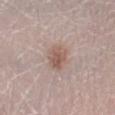Case summary:
- workup · catalogued during a skin exam; not biopsied
- tile lighting · white-light
- lesion size · ≈3.5 mm
- site · the right lower leg
- patient · female, aged 28–32
- TBP lesion metrics · a lesion area of about 7.5 mm², an outline eccentricity of about 0.65 (0 = round, 1 = elongated), and a shape-asymmetry score of about 0.2 (0 = symmetric)
- image · ~15 mm crop, total-body skin-cancer survey